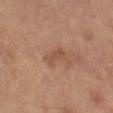No biopsy was performed on this lesion — it was imaged during a full skin examination and was not determined to be concerning.
A roughly 15 mm field-of-view crop from a total-body skin photograph.
Imaged with cross-polarized lighting.
The patient is a male aged around 65.